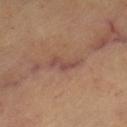Part of a total-body skin-imaging series; this lesion was reviewed on a skin check and was not flagged for biopsy. Automated image analysis of the tile measured a lesion color around L≈47 a*≈21 b*≈24 in CIELAB. It also reported a border-irregularity index near 5/10, a within-lesion color-variation index near 2/10, and radial color variation of about 0.5. And it measured a classifier nevus-likeness of about 0/100 and a detector confidence of about 55 out of 100 that the crop contains a lesion. The lesion is located on the left thigh. The recorded lesion diameter is about 4 mm. A patient in their 60s. A lesion tile, about 15 mm wide, cut from a 3D total-body photograph.